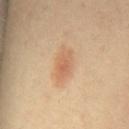Impression:
Recorded during total-body skin imaging; not selected for excision or biopsy.
Acquisition and patient details:
The lesion's longest dimension is about 4 mm. Cropped from a whole-body photographic skin survey; the tile spans about 15 mm. The total-body-photography lesion software estimated a shape eccentricity near 0.85 and a shape-asymmetry score of about 0.2 (0 = symmetric). It also reported a lesion color around L≈61 a*≈20 b*≈34 in CIELAB, roughly 8 lightness units darker than nearby skin, and a lesion-to-skin contrast of about 5.5 (normalized; higher = more distinct). It also reported a detector confidence of about 100 out of 100 that the crop contains a lesion. A female patient, roughly 65 years of age. On the mid back.